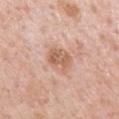Q: Is there a histopathology result?
A: catalogued during a skin exam; not biopsied
Q: How large is the lesion?
A: ~3 mm (longest diameter)
Q: What is the anatomic site?
A: the mid back
Q: Illumination type?
A: white-light illumination
Q: Patient demographics?
A: male, aged approximately 60
Q: What is the imaging modality?
A: ~15 mm tile from a whole-body skin photo
Q: What did automated image analysis measure?
A: a footprint of about 7 mm², an eccentricity of roughly 0.55, and a shape-asymmetry score of about 0.25 (0 = symmetric); an average lesion color of about L≈61 a*≈21 b*≈31 (CIELAB) and a lesion–skin lightness drop of about 10; internal color variation of about 4 on a 0–10 scale